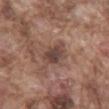The lesion was tiled from a total-body skin photograph and was not biopsied. A male patient, aged 73–77. From the chest. A 15 mm crop from a total-body photograph taken for skin-cancer surveillance. The tile uses white-light illumination. The lesion-visualizer software estimated a footprint of about 6 mm², a shape eccentricity near 0.8, and two-axis asymmetry of about 0.3. The analysis additionally found a lesion color around L≈42 a*≈17 b*≈22 in CIELAB and a lesion–skin lightness drop of about 10.